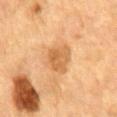Recorded during total-body skin imaging; not selected for excision or biopsy. On the back. This image is a 15 mm lesion crop taken from a total-body photograph. A male patient aged around 85 or older.